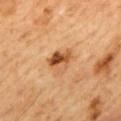{"biopsy_status": "not biopsied; imaged during a skin examination", "image": {"source": "total-body photography crop", "field_of_view_mm": 15}, "automated_metrics": {"shape_asymmetry": 0.2, "border_irregularity_0_10": 2.0, "color_variation_0_10": 10.0, "peripheral_color_asymmetry": 5.0, "lesion_detection_confidence_0_100": 100}, "patient": {"sex": "male", "age_approx": 60}, "site": "mid back", "lesion_size": {"long_diameter_mm_approx": 3.5}}